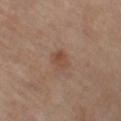Findings:
- notes: imaged on a skin check; not biopsied
- lesion diameter: ~2.5 mm (longest diameter)
- patient: female, approximately 70 years of age
- image: total-body-photography crop, ~15 mm field of view
- automated lesion analysis: a normalized border contrast of about 6; a classifier nevus-likeness of about 20/100 and a lesion-detection confidence of about 100/100
- lighting: cross-polarized
- site: the left lower leg